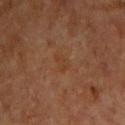Clinical impression:
Captured during whole-body skin photography for melanoma surveillance; the lesion was not biopsied.
Image and clinical context:
A roughly 15 mm field-of-view crop from a total-body skin photograph. Approximately 2.5 mm at its widest. Automated image analysis of the tile measured a border-irregularity rating of about 4/10 and radial color variation of about 0. The patient is a male aged approximately 65. The lesion is located on the chest.